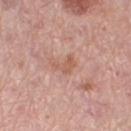No biopsy was performed on this lesion — it was imaged during a full skin examination and was not determined to be concerning. On the right thigh. The lesion's longest dimension is about 2.5 mm. A female patient aged around 45. A region of skin cropped from a whole-body photographic capture, roughly 15 mm wide.